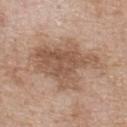notes: no biopsy performed (imaged during a skin exam)
location: the upper back
automated lesion analysis: a lesion color around L≈54 a*≈18 b*≈29 in CIELAB, about 11 CIELAB-L* units darker than the surrounding skin, and a normalized lesion–skin contrast near 7.5; a border-irregularity rating of about 6.5/10, a within-lesion color-variation index near 4/10, and radial color variation of about 1.5; lesion-presence confidence of about 100/100
subject: male, aged 68–72
lesion size: ≈9 mm
image source: 15 mm crop, total-body photography